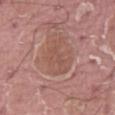Imaged during a routine full-body skin examination; the lesion was not biopsied and no histopathology is available. A close-up tile cropped from a whole-body skin photograph, about 15 mm across. A male patient about 40 years old. An algorithmic analysis of the crop reported a mean CIELAB color near L≈52 a*≈21 b*≈26, roughly 6 lightness units darker than nearby skin, and a lesion-to-skin contrast of about 5 (normalized; higher = more distinct). This is a white-light tile. The lesion is located on the leg.